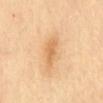Assessment: No biopsy was performed on this lesion — it was imaged during a full skin examination and was not determined to be concerning. Background: Cropped from a whole-body photographic skin survey; the tile spans about 15 mm. A female patient approximately 70 years of age. The tile uses cross-polarized illumination. The recorded lesion diameter is about 4 mm. An algorithmic analysis of the crop reported a lesion area of about 6 mm², an eccentricity of roughly 0.9, and a shape-asymmetry score of about 0.25 (0 = symmetric). It also reported lesion-presence confidence of about 100/100. The lesion is on the front of the torso.